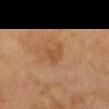{"biopsy_status": "not biopsied; imaged during a skin examination", "lesion_size": {"long_diameter_mm_approx": 3.0}, "automated_metrics": {"area_mm2_approx": 4.0, "eccentricity": 0.7, "border_irregularity_0_10": 4.5, "peripheral_color_asymmetry": 0.5}, "site": "head or neck", "patient": {"sex": "female", "age_approx": 70}, "lighting": "cross-polarized", "image": {"source": "total-body photography crop", "field_of_view_mm": 15}}